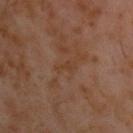biopsy status = catalogued during a skin exam; not biopsied | imaging modality = ~15 mm crop, total-body skin-cancer survey | patient = male, aged 58 to 62 | location = the upper back | TBP lesion metrics = an area of roughly 1.5 mm² and two-axis asymmetry of about 0.55; a lesion color around L≈35 a*≈19 b*≈28 in CIELAB and about 4 CIELAB-L* units darker than the surrounding skin; a peripheral color-asymmetry measure near 0 | lighting = cross-polarized | diameter = ≈2.5 mm.